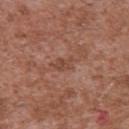biopsy status=total-body-photography surveillance lesion; no biopsy
site=the upper back
patient=male, aged around 45
imaging modality=~15 mm crop, total-body skin-cancer survey